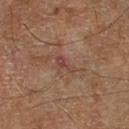Clinical impression: No biopsy was performed on this lesion — it was imaged during a full skin examination and was not determined to be concerning. Clinical summary: A male subject, in their 70s. A roughly 15 mm field-of-view crop from a total-body skin photograph. On the right lower leg.